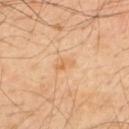Captured during whole-body skin photography for melanoma surveillance; the lesion was not biopsied.
On the upper back.
The subject is a male aged approximately 50.
Cropped from a whole-body photographic skin survey; the tile spans about 15 mm.